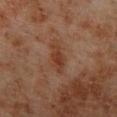Findings:
– workup — total-body-photography surveillance lesion; no biopsy
– imaging modality — 15 mm crop, total-body photography
– subject — male, aged 68–72
– anatomic site — the right lower leg
– lesion size — about 3.5 mm
– lighting — cross-polarized